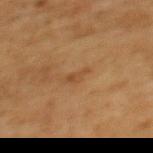Assessment:
Imaged during a routine full-body skin examination; the lesion was not biopsied and no histopathology is available.
Image and clinical context:
Longest diameter approximately 2.5 mm. This is a cross-polarized tile. Cropped from a total-body skin-imaging series; the visible field is about 15 mm. The subject is a female roughly 50 years of age. Automated tile analysis of the lesion measured an average lesion color of about L≈39 a*≈18 b*≈32 (CIELAB), roughly 6 lightness units darker than nearby skin, and a normalized border contrast of about 5.5. And it measured a border-irregularity rating of about 6/10, internal color variation of about 0 on a 0–10 scale, and a peripheral color-asymmetry measure near 0. It also reported an automated nevus-likeness rating near 0 out of 100 and a detector confidence of about 100 out of 100 that the crop contains a lesion. From the upper back.